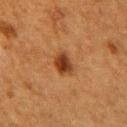Captured during whole-body skin photography for melanoma surveillance; the lesion was not biopsied. A female patient about 50 years old. A 15 mm close-up extracted from a 3D total-body photography capture. Located on the right upper arm. The recorded lesion diameter is about 3 mm.